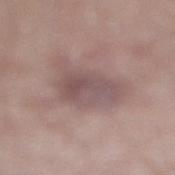The lesion was tiled from a total-body skin photograph and was not biopsied.
The recorded lesion diameter is about 4.5 mm.
A lesion tile, about 15 mm wide, cut from a 3D total-body photograph.
Located on the leg.
The total-body-photography lesion software estimated a border-irregularity index near 3.5/10, a color-variation rating of about 4/10, and radial color variation of about 1.5.
Captured under white-light illumination.
A male subject aged approximately 55.